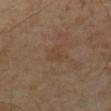workup — no biopsy performed (imaged during a skin exam)
patient — male, about 65 years old
imaging modality — ~15 mm crop, total-body skin-cancer survey
tile lighting — cross-polarized illumination
lesion size — ≈2.5 mm